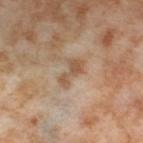Q: Was this lesion biopsied?
A: catalogued during a skin exam; not biopsied
Q: What are the patient's age and sex?
A: female, aged 53 to 57
Q: What is the anatomic site?
A: the left thigh
Q: What is the lesion's diameter?
A: about 3.5 mm
Q: What kind of image is this?
A: ~15 mm crop, total-body skin-cancer survey